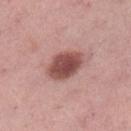Background:
The subject is a female about 50 years old. An algorithmic analysis of the crop reported an outline eccentricity of about 0.8 (0 = round, 1 = elongated). The software also gave a lesion color around L≈49 a*≈25 b*≈23 in CIELAB, a lesion–skin lightness drop of about 16, and a normalized lesion–skin contrast near 10.5. The analysis additionally found a border-irregularity index near 1.5/10, a color-variation rating of about 4/10, and peripheral color asymmetry of about 1. The analysis additionally found lesion-presence confidence of about 100/100. A roughly 15 mm field-of-view crop from a total-body skin photograph. The tile uses white-light illumination. From the left lower leg.